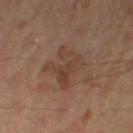A male subject approximately 65 years of age.
A close-up tile cropped from a whole-body skin photograph, about 15 mm across.
Measured at roughly 5 mm in maximum diameter.
The lesion is located on the left leg.
The tile uses cross-polarized illumination.